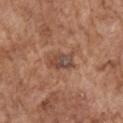The lesion was photographed on a routine skin check and not biopsied; there is no pathology result.
On the right upper arm.
This image is a 15 mm lesion crop taken from a total-body photograph.
An algorithmic analysis of the crop reported a lesion color around L≈46 a*≈20 b*≈27 in CIELAB, about 9 CIELAB-L* units darker than the surrounding skin, and a normalized border contrast of about 7.5. The software also gave border irregularity of about 2.5 on a 0–10 scale, internal color variation of about 9.5 on a 0–10 scale, and peripheral color asymmetry of about 3.5.
This is a white-light tile.
A male subject approximately 75 years of age.
Longest diameter approximately 3.5 mm.